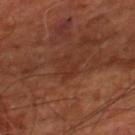Assessment:
Part of a total-body skin-imaging series; this lesion was reviewed on a skin check and was not flagged for biopsy.
Background:
Cropped from a whole-body photographic skin survey; the tile spans about 15 mm. Captured under cross-polarized illumination. Located on the right lower leg. The subject is in their mid-60s.